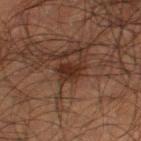Clinical impression: This lesion was catalogued during total-body skin photography and was not selected for biopsy. Acquisition and patient details: The lesion is located on the left thigh. A male subject approximately 50 years of age. A lesion tile, about 15 mm wide, cut from a 3D total-body photograph. This is a cross-polarized tile. Longest diameter approximately 3.5 mm.